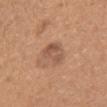follow-up: catalogued during a skin exam; not biopsied | subject: female, aged 38 to 42 | image source: ~15 mm tile from a whole-body skin photo | site: the chest | lighting: white-light illumination.